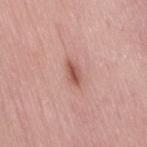{
  "biopsy_status": "not biopsied; imaged during a skin examination",
  "image": {
    "source": "total-body photography crop",
    "field_of_view_mm": 15
  },
  "patient": {
    "sex": "female",
    "age_approx": 50
  },
  "automated_metrics": {
    "area_mm2_approx": 4.0,
    "shape_asymmetry": 0.25,
    "border_irregularity_0_10": 2.5,
    "color_variation_0_10": 3.0,
    "peripheral_color_asymmetry": 1.0
  },
  "site": "right thigh"
}